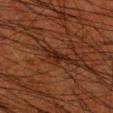This lesion was catalogued during total-body skin photography and was not selected for biopsy. A 15 mm close-up extracted from a 3D total-body photography capture. Captured under cross-polarized illumination. Longest diameter approximately 2.5 mm. Automated image analysis of the tile measured a lesion area of about 2.5 mm², a shape eccentricity near 0.9, and a symmetry-axis asymmetry near 0.4. The lesion is on the right upper arm. The subject is a male aged 48 to 52.